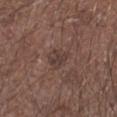Notes:
– follow-up · no biopsy performed (imaged during a skin exam)
– patient · male, aged approximately 65
– lighting · white-light illumination
– size · ≈3 mm
– imaging modality · ~15 mm crop, total-body skin-cancer survey
– location · the left upper arm